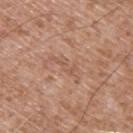Notes:
* follow-up: no biopsy performed (imaged during a skin exam)
* illumination: white-light illumination
* patient: male, aged around 55
* location: the upper back
* image: ~15 mm tile from a whole-body skin photo